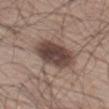Findings:
– biopsy status — total-body-photography surveillance lesion; no biopsy
– image source — 15 mm crop, total-body photography
– tile lighting — white-light
– lesion diameter — ≈6 mm
– anatomic site — the left thigh
– subject — male, approximately 60 years of age
– automated lesion analysis — a border-irregularity rating of about 1.5/10, internal color variation of about 4.5 on a 0–10 scale, and radial color variation of about 1.5; a nevus-likeness score of about 65/100 and a lesion-detection confidence of about 100/100